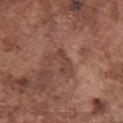<record>
  <biopsy_status>not biopsied; imaged during a skin examination</biopsy_status>
  <image>
    <source>total-body photography crop</source>
    <field_of_view_mm>15</field_of_view_mm>
  </image>
  <lesion_size>
    <long_diameter_mm_approx>3.5</long_diameter_mm_approx>
  </lesion_size>
  <patient>
    <sex>male</sex>
    <age_approx>75</age_approx>
  </patient>
  <lighting>white-light</lighting>
  <site>chest</site>
</record>